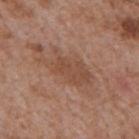Cropped from a total-body skin-imaging series; the visible field is about 15 mm.
Captured under white-light illumination.
The lesion is on the mid back.
A male subject in their mid- to late 60s.
The recorded lesion diameter is about 6.5 mm.